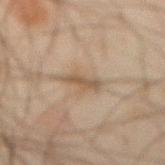Part of a total-body skin-imaging series; this lesion was reviewed on a skin check and was not flagged for biopsy. This is a cross-polarized tile. The lesion is on the abdomen. A male patient aged 43–47. Cropped from a whole-body photographic skin survey; the tile spans about 15 mm.